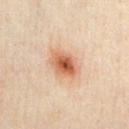workup: no biopsy performed (imaged during a skin exam); image: total-body-photography crop, ~15 mm field of view; illumination: cross-polarized illumination; anatomic site: the chest; patient: male, in their mid- to late 30s.